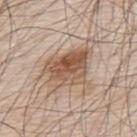biopsy status=catalogued during a skin exam; not biopsied | site=the upper back | image=15 mm crop, total-body photography | tile lighting=white-light illumination | subject=male, aged approximately 80 | diameter=≈6 mm.